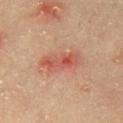Acquisition and patient details: Measured at roughly 4.5 mm in maximum diameter. Imaged with cross-polarized lighting. Cropped from a whole-body photographic skin survey; the tile spans about 15 mm. The total-body-photography lesion software estimated a footprint of about 8 mm² and an eccentricity of roughly 0.9. It also reported a border-irregularity rating of about 3.5/10, a within-lesion color-variation index near 8.5/10, and a peripheral color-asymmetry measure near 3. And it measured a nevus-likeness score of about 0/100 and lesion-presence confidence of about 100/100. A male subject aged approximately 65. The lesion is on the chest.